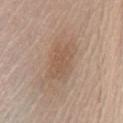– biopsy status · catalogued during a skin exam; not biopsied
– patient · male, aged 63–67
– site · the chest
– automated metrics · a lesion area of about 11 mm², a shape eccentricity near 0.85, and a shape-asymmetry score of about 0.25 (0 = symmetric); an average lesion color of about L≈55 a*≈16 b*≈29 (CIELAB) and a normalized border contrast of about 5.5; a border-irregularity rating of about 3.5/10 and a color-variation rating of about 2.5/10
– lighting · white-light
– size · ≈5 mm
– acquisition · 15 mm crop, total-body photography